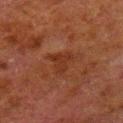{"lighting": "cross-polarized", "image": {"source": "total-body photography crop", "field_of_view_mm": 15}, "site": "left lower leg", "automated_metrics": {"area_mm2_approx": 5.0, "eccentricity": 0.4, "vs_skin_darker_L": 5.0, "vs_skin_contrast_norm": 6.0, "nevus_likeness_0_100": 0, "lesion_detection_confidence_0_100": 100}, "patient": {"sex": "male", "age_approx": 80}, "lesion_size": {"long_diameter_mm_approx": 3.0}}